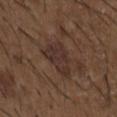The lesion-visualizer software estimated an eccentricity of roughly 0.75 and a shape-asymmetry score of about 0.3 (0 = symmetric). It also reported a border-irregularity index near 3.5/10 and a peripheral color-asymmetry measure near 1. The analysis additionally found a classifier nevus-likeness of about 0/100 and a detector confidence of about 95 out of 100 that the crop contains a lesion. A male subject roughly 50 years of age. Captured under white-light illumination. Measured at roughly 4.5 mm in maximum diameter. A 15 mm close-up extracted from a 3D total-body photography capture. From the chest.